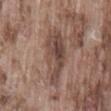workup: total-body-photography surveillance lesion; no biopsy
image source: ~15 mm crop, total-body skin-cancer survey
anatomic site: the lower back
patient: male, aged approximately 75
lesion size: ≈6.5 mm
automated metrics: a footprint of about 17 mm², a shape eccentricity near 0.85, and two-axis asymmetry of about 0.25; an automated nevus-likeness rating near 5 out of 100 and a detector confidence of about 65 out of 100 that the crop contains a lesion
lighting: white-light illumination